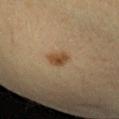Impression: Recorded during total-body skin imaging; not selected for excision or biopsy. Background: The tile uses cross-polarized illumination. A female patient aged around 30. A lesion tile, about 15 mm wide, cut from a 3D total-body photograph. Longest diameter approximately 2.5 mm. From the right forearm.